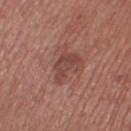<record>
  <biopsy_status>not biopsied; imaged during a skin examination</biopsy_status>
  <site>right thigh</site>
  <automated_metrics>
    <cielab_L>44</cielab_L>
    <cielab_a>23</cielab_a>
    <cielab_b>24</cielab_b>
    <vs_skin_darker_L>8.0</vs_skin_darker_L>
    <vs_skin_contrast_norm>6.5</vs_skin_contrast_norm>
    <border_irregularity_0_10>5.0</border_irregularity_0_10>
    <color_variation_0_10>2.5</color_variation_0_10>
    <peripheral_color_asymmetry>0.5</peripheral_color_asymmetry>
    <nevus_likeness_0_100>5</nevus_likeness_0_100>
    <lesion_detection_confidence_0_100>100</lesion_detection_confidence_0_100>
  </automated_metrics>
  <lighting>white-light</lighting>
  <image>
    <source>total-body photography crop</source>
    <field_of_view_mm>15</field_of_view_mm>
  </image>
  <patient>
    <sex>male</sex>
    <age_approx>70</age_approx>
  </patient>
</record>